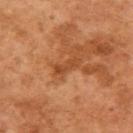follow-up: catalogued during a skin exam; not biopsied | lesion size: about 3.5 mm | illumination: cross-polarized illumination | image source: 15 mm crop, total-body photography | site: the right upper arm | patient: female, aged approximately 60.